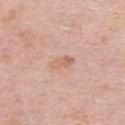Image and clinical context:
Longest diameter approximately 3 mm. The subject is a male aged 73–77. From the upper back. A 15 mm close-up extracted from a 3D total-body photography capture.